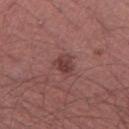Impression:
The lesion was tiled from a total-body skin photograph and was not biopsied.
Acquisition and patient details:
The subject is a male roughly 45 years of age. A 15 mm close-up tile from a total-body photography series done for melanoma screening. The lesion is on the right thigh.